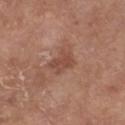Imaged during a routine full-body skin examination; the lesion was not biopsied and no histopathology is available. A male subject, aged around 70. Located on the left lower leg. A roughly 15 mm field-of-view crop from a total-body skin photograph.